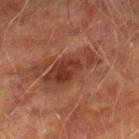Impression: Recorded during total-body skin imaging; not selected for excision or biopsy. Image and clinical context: The lesion is on the right lower leg. A 15 mm crop from a total-body photograph taken for skin-cancer surveillance. A male subject, aged 73–77. Imaged with cross-polarized lighting.